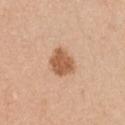| key | value |
|---|---|
| workup | catalogued during a skin exam; not biopsied |
| anatomic site | the chest |
| subject | female, aged around 45 |
| acquisition | total-body-photography crop, ~15 mm field of view |
| lesion size | about 3.5 mm |
| image-analysis metrics | a footprint of about 8 mm², a shape eccentricity near 0.55, and two-axis asymmetry of about 0.2; a border-irregularity rating of about 1.5/10, a within-lesion color-variation index near 2.5/10, and a peripheral color-asymmetry measure near 1 |